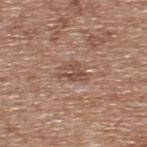biopsy status = no biopsy performed (imaged during a skin exam)